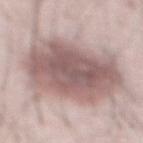Imaged during a routine full-body skin examination; the lesion was not biopsied and no histopathology is available.
Located on the abdomen.
The subject is a male aged approximately 65.
A 15 mm crop from a total-body photograph taken for skin-cancer surveillance.
Captured under white-light illumination.
Longest diameter approximately 10.5 mm.